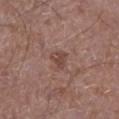Captured during whole-body skin photography for melanoma surveillance; the lesion was not biopsied.
The lesion is located on the left lower leg.
A close-up tile cropped from a whole-body skin photograph, about 15 mm across.
The tile uses white-light illumination.
The patient is a male approximately 45 years of age.
Approximately 2.5 mm at its widest.
The total-body-photography lesion software estimated an area of roughly 4 mm², a shape eccentricity near 0.5, and a symmetry-axis asymmetry near 0.45. The software also gave a border-irregularity index near 4/10 and a color-variation rating of about 1.5/10. The software also gave an automated nevus-likeness rating near 0 out of 100 and a lesion-detection confidence of about 100/100.